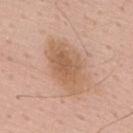{"biopsy_status": "not biopsied; imaged during a skin examination", "image": {"source": "total-body photography crop", "field_of_view_mm": 15}, "patient": {"sex": "male", "age_approx": 55}, "lighting": "white-light", "lesion_size": {"long_diameter_mm_approx": 6.5}, "site": "upper back"}